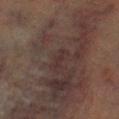Part of a total-body skin-imaging series; this lesion was reviewed on a skin check and was not flagged for biopsy. Located on the left lower leg. This is a cross-polarized tile. A male patient, aged 68 to 72. Measured at roughly 2.5 mm in maximum diameter. A roughly 15 mm field-of-view crop from a total-body skin photograph.